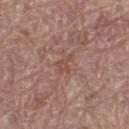{
  "lighting": "white-light",
  "site": "left thigh",
  "image": {
    "source": "total-body photography crop",
    "field_of_view_mm": 15
  },
  "patient": {
    "sex": "male",
    "age_approx": 65
  }
}